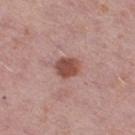Clinical impression:
Imaged during a routine full-body skin examination; the lesion was not biopsied and no histopathology is available.
Clinical summary:
The lesion-visualizer software estimated a border-irregularity rating of about 1/10 and radial color variation of about 0.5. And it measured a classifier nevus-likeness of about 90/100 and a detector confidence of about 100 out of 100 that the crop contains a lesion. The lesion is on the right thigh. A female patient aged approximately 50. A lesion tile, about 15 mm wide, cut from a 3D total-body photograph.